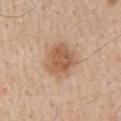Cropped from a whole-body photographic skin survey; the tile spans about 15 mm.
A male patient, aged around 60.
Approximately 4 mm at its widest.
This is a white-light tile.
The lesion is on the mid back.